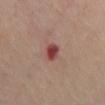workup: no biopsy performed (imaged during a skin exam)
tile lighting: cross-polarized
automated lesion analysis: a border-irregularity index near 2/10, a within-lesion color-variation index near 3.5/10, and a peripheral color-asymmetry measure near 1; a classifier nevus-likeness of about 0/100
location: the mid back
size: ~2.5 mm (longest diameter)
imaging modality: ~15 mm tile from a whole-body skin photo
subject: female, approximately 60 years of age